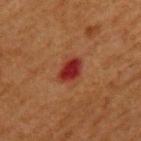Clinical impression: Part of a total-body skin-imaging series; this lesion was reviewed on a skin check and was not flagged for biopsy.